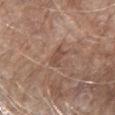notes: total-body-photography surveillance lesion; no biopsy
lighting: white-light
diameter: ≈3 mm
location: the right upper arm
image source: ~15 mm tile from a whole-body skin photo
patient: female, aged approximately 85 or older
TBP lesion metrics: a lesion color around L≈48 a*≈19 b*≈27 in CIELAB, roughly 7 lightness units darker than nearby skin, and a normalized lesion–skin contrast near 6; a nevus-likeness score of about 0/100 and lesion-presence confidence of about 100/100